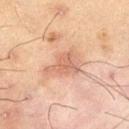{"biopsy_status": "not biopsied; imaged during a skin examination", "image": {"source": "total-body photography crop", "field_of_view_mm": 15}, "lesion_size": {"long_diameter_mm_approx": 5.0}, "patient": {"sex": "male", "age_approx": 70}, "site": "left thigh", "automated_metrics": {"border_irregularity_0_10": 4.5, "color_variation_0_10": 2.5, "peripheral_color_asymmetry": 0.5, "nevus_likeness_0_100": 30, "lesion_detection_confidence_0_100": 100}}